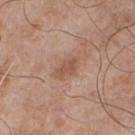lighting = white-light
diameter = ~2.5 mm (longest diameter)
automated lesion analysis = peripheral color asymmetry of about 0.5
body site = the chest
image = total-body-photography crop, ~15 mm field of view
subject = male, aged approximately 65